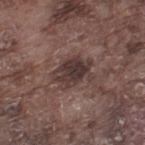Findings:
* notes · catalogued during a skin exam; not biopsied
* automated lesion analysis · an outline eccentricity of about 0.7 (0 = round, 1 = elongated) and a shape-asymmetry score of about 0.35 (0 = symmetric)
* diameter · ~4.5 mm (longest diameter)
* site · the left thigh
* lighting · white-light illumination
* image source · 15 mm crop, total-body photography
* patient · male, aged around 75